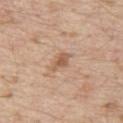Assessment: Captured during whole-body skin photography for melanoma surveillance; the lesion was not biopsied. Context: The lesion is located on the abdomen. This image is a 15 mm lesion crop taken from a total-body photograph. A male subject, approximately 70 years of age.